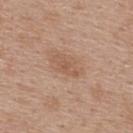Findings:
– workup · imaged on a skin check; not biopsied
– lighting · white-light illumination
– site · the upper back
– automated metrics · a lesion area of about 3.5 mm², an eccentricity of roughly 0.9, and two-axis asymmetry of about 0.35; roughly 7 lightness units darker than nearby skin and a lesion-to-skin contrast of about 5 (normalized; higher = more distinct)
– lesion size · ≈3 mm
– image · 15 mm crop, total-body photography
– subject · female, aged 38 to 42